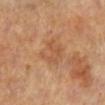Imaged during a routine full-body skin examination; the lesion was not biopsied and no histopathology is available.
Measured at roughly 3.5 mm in maximum diameter.
A female patient, in their mid-60s.
An algorithmic analysis of the crop reported a lesion area of about 7 mm², an eccentricity of roughly 0.65, and a symmetry-axis asymmetry near 0.3. The software also gave border irregularity of about 5 on a 0–10 scale and a within-lesion color-variation index near 3/10.
On the right leg.
A roughly 15 mm field-of-view crop from a total-body skin photograph.